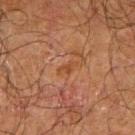Impression: No biopsy was performed on this lesion — it was imaged during a full skin examination and was not determined to be concerning. Clinical summary: A lesion tile, about 15 mm wide, cut from a 3D total-body photograph. The lesion is located on the right upper arm. A male subject, about 70 years old.